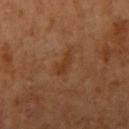Case summary:
– follow-up · no biopsy performed (imaged during a skin exam)
– body site · the right upper arm
– acquisition · ~15 mm crop, total-body skin-cancer survey
– subject · male, about 60 years old